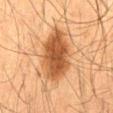Captured during whole-body skin photography for melanoma surveillance; the lesion was not biopsied. A male patient in their mid- to late 30s. Measured at roughly 7 mm in maximum diameter. Cropped from a total-body skin-imaging series; the visible field is about 15 mm. Located on the back. Imaged with cross-polarized lighting.